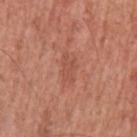{
  "biopsy_status": "not biopsied; imaged during a skin examination",
  "lesion_size": {
    "long_diameter_mm_approx": 3.5
  },
  "automated_metrics": {
    "area_mm2_approx": 5.0,
    "eccentricity": 0.8,
    "shape_asymmetry": 0.35,
    "color_variation_0_10": 1.5,
    "peripheral_color_asymmetry": 0.5
  },
  "site": "right upper arm",
  "patient": {
    "sex": "male",
    "age_approx": 45
  },
  "image": {
    "source": "total-body photography crop",
    "field_of_view_mm": 15
  },
  "lighting": "white-light"
}